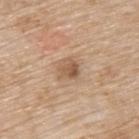automated metrics=border irregularity of about 3 on a 0–10 scale, a color-variation rating of about 4/10, and radial color variation of about 1.5; a classifier nevus-likeness of about 15/100 and a lesion-detection confidence of about 100/100
patient=female, in their mid- to late 70s
image=total-body-photography crop, ~15 mm field of view
anatomic site=the upper back